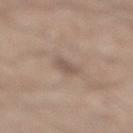Q: How was this image acquired?
A: 15 mm crop, total-body photography
Q: What are the patient's age and sex?
A: male, in their mid- to late 60s
Q: How was the tile lit?
A: white-light
Q: Lesion location?
A: the right lower leg
Q: Lesion size?
A: ~3.5 mm (longest diameter)
Q: Automated lesion metrics?
A: a lesion area of about 5.5 mm², a shape eccentricity near 0.7, and a symmetry-axis asymmetry near 0.3; a classifier nevus-likeness of about 0/100 and a detector confidence of about 100 out of 100 that the crop contains a lesion